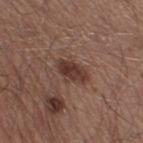Imaged during a routine full-body skin examination; the lesion was not biopsied and no histopathology is available.
Measured at roughly 4 mm in maximum diameter.
Located on the right thigh.
A 15 mm crop from a total-body photograph taken for skin-cancer surveillance.
A male patient, aged 38–42.
The tile uses white-light illumination.
Automated tile analysis of the lesion measured a footprint of about 6.5 mm², a shape eccentricity near 0.85, and a symmetry-axis asymmetry near 0.2. It also reported a mean CIELAB color near L≈36 a*≈19 b*≈23, roughly 11 lightness units darker than nearby skin, and a normalized border contrast of about 9.5. And it measured internal color variation of about 3.5 on a 0–10 scale and a peripheral color-asymmetry measure near 1.